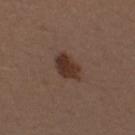biopsy_status: not biopsied; imaged during a skin examination
lighting: white-light
image:
  source: total-body photography crop
  field_of_view_mm: 15
lesion_size:
  long_diameter_mm_approx: 3.5
patient:
  sex: male
  age_approx: 30
site: left upper arm